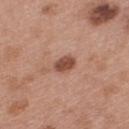biopsy status — imaged on a skin check; not biopsied
location — the upper back
lesion diameter — ≈3 mm
image — total-body-photography crop, ~15 mm field of view
patient — female, about 40 years old
lighting — white-light